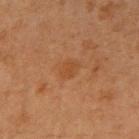Impression:
No biopsy was performed on this lesion — it was imaged during a full skin examination and was not determined to be concerning.
Acquisition and patient details:
The tile uses cross-polarized illumination. A close-up tile cropped from a whole-body skin photograph, about 15 mm across. A female patient, aged 38–42. Measured at roughly 2.5 mm in maximum diameter. The lesion is on the right upper arm.